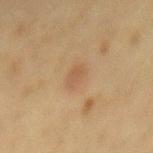Recorded during total-body skin imaging; not selected for excision or biopsy. This image is a 15 mm lesion crop taken from a total-body photograph. Measured at roughly 3 mm in maximum diameter. From the mid back. Imaged with cross-polarized lighting. The subject is a female approximately 50 years of age.